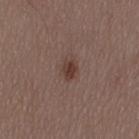Notes:
• follow-up · catalogued during a skin exam; not biopsied
• subject · male, roughly 50 years of age
• automated metrics · an area of roughly 4.5 mm², an outline eccentricity of about 0.7 (0 = round, 1 = elongated), and a symmetry-axis asymmetry near 0.2; a nevus-likeness score of about 95/100 and lesion-presence confidence of about 100/100
• size · about 2.5 mm
• anatomic site · the lower back
• illumination · white-light
• acquisition · ~15 mm tile from a whole-body skin photo